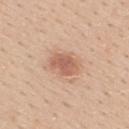A male subject, aged approximately 30. The lesion is on the upper back. Captured under white-light illumination. A 15 mm close-up tile from a total-body photography series done for melanoma screening.